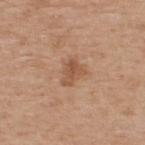The lesion was photographed on a routine skin check and not biopsied; there is no pathology result. About 3 mm across. A male subject in their mid-60s. Imaged with white-light lighting. Cropped from a total-body skin-imaging series; the visible field is about 15 mm. Located on the back. Automated tile analysis of the lesion measured internal color variation of about 3 on a 0–10 scale and peripheral color asymmetry of about 1. The software also gave a classifier nevus-likeness of about 15/100 and lesion-presence confidence of about 100/100.